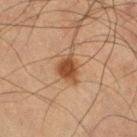follow-up: imaged on a skin check; not biopsied
image source: ~15 mm tile from a whole-body skin photo
anatomic site: the left thigh
illumination: cross-polarized
automated metrics: an area of roughly 8 mm², a shape eccentricity near 0.8, and a symmetry-axis asymmetry near 0.35; a mean CIELAB color near L≈39 a*≈18 b*≈29, about 10 CIELAB-L* units darker than the surrounding skin, and a normalized border contrast of about 9; an automated nevus-likeness rating near 95 out of 100 and a lesion-detection confidence of about 100/100
subject: male, aged approximately 65